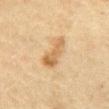The lesion was photographed on a routine skin check and not biopsied; there is no pathology result.
The lesion is on the abdomen.
The subject is a female aged 53–57.
A 15 mm close-up extracted from a 3D total-body photography capture.